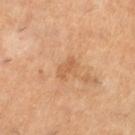Imaged during a routine full-body skin examination; the lesion was not biopsied and no histopathology is available. Imaged with cross-polarized lighting. About 2.5 mm across. A 15 mm close-up extracted from a 3D total-body photography capture. On the left lower leg. Automated tile analysis of the lesion measured a normalized border contrast of about 5. The analysis additionally found a border-irregularity rating of about 3/10, a within-lesion color-variation index near 0/10, and a peripheral color-asymmetry measure near 0. It also reported a nevus-likeness score of about 0/100 and a lesion-detection confidence of about 100/100. A female patient aged approximately 55.